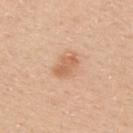biopsy status — imaged on a skin check; not biopsied | anatomic site — the upper back | imaging modality — total-body-photography crop, ~15 mm field of view | subject — male, in their 30s.